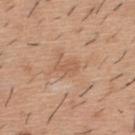{"biopsy_status": "not biopsied; imaged during a skin examination", "lighting": "white-light", "lesion_size": {"long_diameter_mm_approx": 3.0}, "site": "back", "automated_metrics": {"border_irregularity_0_10": 3.5, "color_variation_0_10": 0.5, "peripheral_color_asymmetry": 0.0, "nevus_likeness_0_100": 0}, "image": {"source": "total-body photography crop", "field_of_view_mm": 15}, "patient": {"sex": "male", "age_approx": 40}}